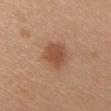| feature | finding |
|---|---|
| workup | imaged on a skin check; not biopsied |
| subject | female, roughly 45 years of age |
| TBP lesion metrics | about 10 CIELAB-L* units darker than the surrounding skin and a normalized border contrast of about 7; radial color variation of about 1; a nevus-likeness score of about 95/100 and a detector confidence of about 100 out of 100 that the crop contains a lesion |
| image source | 15 mm crop, total-body photography |
| lesion size | about 3.5 mm |
| tile lighting | white-light |
| body site | the chest |